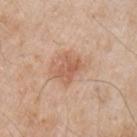Notes:
• follow-up — catalogued during a skin exam; not biopsied
• anatomic site — the left upper arm
• imaging modality — total-body-photography crop, ~15 mm field of view
• illumination — white-light illumination
• size — about 3 mm
• automated metrics — a lesion area of about 6.5 mm² and a shape eccentricity near 0.7; an average lesion color of about L≈59 a*≈22 b*≈32 (CIELAB), about 9 CIELAB-L* units darker than the surrounding skin, and a normalized lesion–skin contrast near 6.5; internal color variation of about 3.5 on a 0–10 scale and peripheral color asymmetry of about 1.5
• patient — male, aged 63 to 67